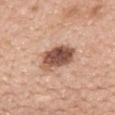  biopsy_status: not biopsied; imaged during a skin examination
  site: mid back
  lighting: white-light
  automated_metrics:
    area_mm2_approx: 11.0
    cielab_L: 52
    cielab_a: 22
    cielab_b: 28
    vs_skin_darker_L: 18.0
    vs_skin_contrast_norm: 11.5
    border_irregularity_0_10: 2.5
    color_variation_0_10: 7.0
    peripheral_color_asymmetry: 2.5
  patient:
    sex: female
    age_approx: 60
  image:
    source: total-body photography crop
    field_of_view_mm: 15
  lesion_size:
    long_diameter_mm_approx: 5.0